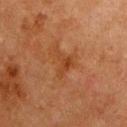Impression: Part of a total-body skin-imaging series; this lesion was reviewed on a skin check and was not flagged for biopsy. Clinical summary: Cropped from a whole-body photographic skin survey; the tile spans about 15 mm. The patient is a female aged approximately 50. Located on the front of the torso.